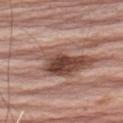Recorded during total-body skin imaging; not selected for excision or biopsy. Cropped from a whole-body photographic skin survey; the tile spans about 15 mm. The total-body-photography lesion software estimated a footprint of about 20 mm², an outline eccentricity of about 0.9 (0 = round, 1 = elongated), and a symmetry-axis asymmetry near 0.45. The software also gave a lesion color around L≈48 a*≈21 b*≈25 in CIELAB and a lesion-to-skin contrast of about 10 (normalized; higher = more distinct). It also reported a classifier nevus-likeness of about 15/100 and a lesion-detection confidence of about 55/100. From the arm. A male patient in their mid-60s. About 10 mm across. The tile uses white-light illumination.